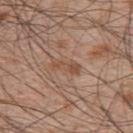Part of a total-body skin-imaging series; this lesion was reviewed on a skin check and was not flagged for biopsy.
Located on the upper back.
Longest diameter approximately 3.5 mm.
A male patient aged 48 to 52.
A 15 mm crop from a total-body photograph taken for skin-cancer surveillance.
The total-body-photography lesion software estimated a shape eccentricity near 0.95 and a shape-asymmetry score of about 0.35 (0 = symmetric). The software also gave a mean CIELAB color near L≈50 a*≈19 b*≈29, a lesion–skin lightness drop of about 7, and a normalized lesion–skin contrast near 6. And it measured a detector confidence of about 100 out of 100 that the crop contains a lesion.